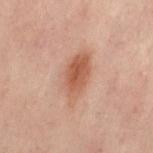notes — imaged on a skin check; not biopsied | acquisition — ~15 mm tile from a whole-body skin photo | size — ~5.5 mm (longest diameter) | location — the lower back | image-analysis metrics — an average lesion color of about L≈47 a*≈20 b*≈26 (CIELAB), a lesion–skin lightness drop of about 9, and a lesion-to-skin contrast of about 7 (normalized; higher = more distinct); a detector confidence of about 100 out of 100 that the crop contains a lesion | subject — female, about 55 years old | illumination — cross-polarized illumination.